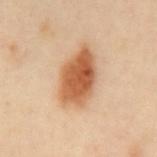Imaged during a routine full-body skin examination; the lesion was not biopsied and no histopathology is available.
Located on the mid back.
This is a cross-polarized tile.
Cropped from a whole-body photographic skin survey; the tile spans about 15 mm.
A male subject, in their mid- to late 60s.
Longest diameter approximately 6.5 mm.